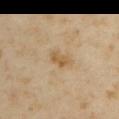Recorded during total-body skin imaging; not selected for excision or biopsy.
A female subject, aged approximately 35.
Automated image analysis of the tile measured an area of roughly 3 mm², an eccentricity of roughly 0.9, and two-axis asymmetry of about 0.25. It also reported a lesion color around L≈54 a*≈15 b*≈36 in CIELAB, about 8 CIELAB-L* units darker than the surrounding skin, and a normalized border contrast of about 7. And it measured lesion-presence confidence of about 100/100.
Approximately 3 mm at its widest.
Captured under cross-polarized illumination.
On the right upper arm.
A region of skin cropped from a whole-body photographic capture, roughly 15 mm wide.